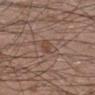| key | value |
|---|---|
| workup | no biopsy performed (imaged during a skin exam) |
| site | the left lower leg |
| subject | male, in their 30s |
| automated metrics | about 7 CIELAB-L* units darker than the surrounding skin and a normalized lesion–skin contrast near 5.5; a nevus-likeness score of about 0/100 and a lesion-detection confidence of about 100/100 |
| illumination | white-light illumination |
| diameter | about 3 mm |
| acquisition | total-body-photography crop, ~15 mm field of view |